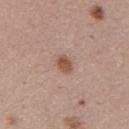body site=the mid back
subject=male, about 55 years old
imaging modality=15 mm crop, total-body photography
diameter=~2.5 mm (longest diameter)
lighting=white-light